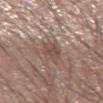Acquisition and patient details:
Longest diameter approximately 4 mm. Cropped from a total-body skin-imaging series; the visible field is about 15 mm. Imaged with white-light lighting. From the left forearm. A male patient, aged around 30.